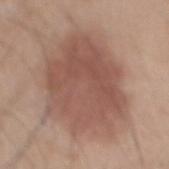Clinical impression:
Recorded during total-body skin imaging; not selected for excision or biopsy.
Image and clinical context:
Automated tile analysis of the lesion measured an area of roughly 55 mm², an outline eccentricity of about 0.65 (0 = round, 1 = elongated), and a shape-asymmetry score of about 0.15 (0 = symmetric). Approximately 10 mm at its widest. On the mid back. The tile uses white-light illumination. A male patient aged approximately 45. Cropped from a whole-body photographic skin survey; the tile spans about 15 mm.